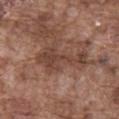| key | value |
|---|---|
| workup | no biopsy performed (imaged during a skin exam) |
| body site | the abdomen |
| lighting | white-light |
| imaging modality | total-body-photography crop, ~15 mm field of view |
| lesion size | ~6.5 mm (longest diameter) |
| subject | male, aged around 75 |
| image-analysis metrics | an area of roughly 10 mm² and an outline eccentricity of about 0.95 (0 = round, 1 = elongated); an average lesion color of about L≈42 a*≈19 b*≈26 (CIELAB), about 9 CIELAB-L* units darker than the surrounding skin, and a normalized lesion–skin contrast near 7.5 |